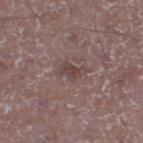The lesion was tiled from a total-body skin photograph and was not biopsied.
The patient is a male aged 48–52.
Captured under white-light illumination.
On the right lower leg.
The lesion's longest dimension is about 4 mm.
A 15 mm close-up tile from a total-body photography series done for melanoma screening.